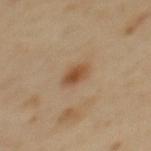Part of a total-body skin-imaging series; this lesion was reviewed on a skin check and was not flagged for biopsy.
The lesion-visualizer software estimated a mean CIELAB color near L≈50 a*≈20 b*≈34 and a lesion–skin lightness drop of about 10. The analysis additionally found a border-irregularity index near 1.5/10, a color-variation rating of about 3.5/10, and a peripheral color-asymmetry measure near 1. The analysis additionally found a classifier nevus-likeness of about 95/100 and lesion-presence confidence of about 100/100.
Imaged with cross-polarized lighting.
From the upper back.
A female subject, roughly 40 years of age.
A 15 mm close-up tile from a total-body photography series done for melanoma screening.